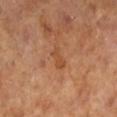No biopsy was performed on this lesion — it was imaged during a full skin examination and was not determined to be concerning. On the left lower leg. The patient is a female in their mid-60s. A close-up tile cropped from a whole-body skin photograph, about 15 mm across.